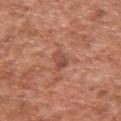Clinical impression: Captured during whole-body skin photography for melanoma surveillance; the lesion was not biopsied. Background: Longest diameter approximately 2.5 mm. Cropped from a whole-body photographic skin survey; the tile spans about 15 mm. A male patient about 45 years old. Located on the arm. The lesion-visualizer software estimated a footprint of about 3.5 mm² and a shape-asymmetry score of about 0.25 (0 = symmetric). And it measured a border-irregularity rating of about 2.5/10, a within-lesion color-variation index near 3.5/10, and peripheral color asymmetry of about 1.5. And it measured a classifier nevus-likeness of about 0/100 and a detector confidence of about 100 out of 100 that the crop contains a lesion. Imaged with white-light lighting.